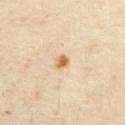Recorded during total-body skin imaging; not selected for excision or biopsy. An algorithmic analysis of the crop reported border irregularity of about 1.5 on a 0–10 scale, internal color variation of about 3.5 on a 0–10 scale, and peripheral color asymmetry of about 1. A region of skin cropped from a whole-body photographic capture, roughly 15 mm wide. The patient is a female aged 38–42. The lesion is located on the abdomen.